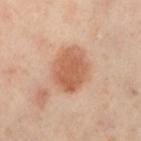Notes:
• workup · catalogued during a skin exam; not biopsied
• subject · female, roughly 60 years of age
• lesion size · ≈4.5 mm
• body site · the right leg
• image · ~15 mm crop, total-body skin-cancer survey
• illumination · cross-polarized